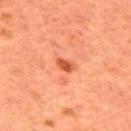Notes:
- patient: male, aged 63 to 67
- body site: the upper back
- image source: total-body-photography crop, ~15 mm field of view
- illumination: cross-polarized